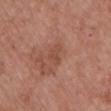follow-up: total-body-photography surveillance lesion; no biopsy
automated lesion analysis: a lesion area of about 3.5 mm², an eccentricity of roughly 0.7, and a shape-asymmetry score of about 0.55 (0 = symmetric); an automated nevus-likeness rating near 0 out of 100 and a detector confidence of about 100 out of 100 that the crop contains a lesion
image source: 15 mm crop, total-body photography
anatomic site: the front of the torso
lesion size: about 2.5 mm
tile lighting: white-light illumination
patient: male, in their mid-50s